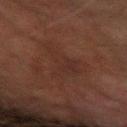Impression:
This lesion was catalogued during total-body skin photography and was not selected for biopsy.
Context:
The patient is a male in their mid- to late 70s. The tile uses cross-polarized illumination. Located on the left forearm. About 6 mm across. A close-up tile cropped from a whole-body skin photograph, about 15 mm across.